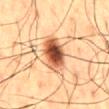A male patient aged approximately 60.
Approximately 5.5 mm at its widest.
Cropped from a whole-body photographic skin survey; the tile spans about 15 mm.
Captured under cross-polarized illumination.
On the mid back.